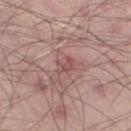The lesion was photographed on a routine skin check and not biopsied; there is no pathology result. The lesion's longest dimension is about 2.5 mm. This image is a 15 mm lesion crop taken from a total-body photograph. The lesion is on the right thigh. A male subject in their mid- to late 40s. The tile uses white-light illumination.